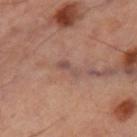biopsy status = total-body-photography surveillance lesion; no biopsy
anatomic site = the leg
tile lighting = cross-polarized illumination
image = ~15 mm crop, total-body skin-cancer survey